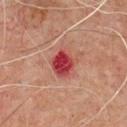notes: total-body-photography surveillance lesion; no biopsy
illumination: cross-polarized illumination
TBP lesion metrics: a border-irregularity rating of about 1/10
image: 15 mm crop, total-body photography
site: the chest
lesion size: ~3.5 mm (longest diameter)
subject: male, in their 60s